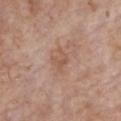Notes:
* biopsy status — total-body-photography surveillance lesion; no biopsy
* body site — the chest
* imaging modality — ~15 mm crop, total-body skin-cancer survey
* subject — female, in their mid-80s or older
* lesion size — about 3 mm
* tile lighting — white-light illumination